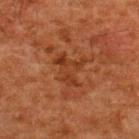The lesion was photographed on a routine skin check and not biopsied; there is no pathology result. Longest diameter approximately 4 mm. A region of skin cropped from a whole-body photographic capture, roughly 15 mm wide. A male patient, aged approximately 60. Imaged with cross-polarized lighting. Located on the upper back.